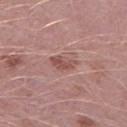{
  "biopsy_status": "not biopsied; imaged during a skin examination",
  "automated_metrics": {
    "cielab_L": 51,
    "cielab_a": 23,
    "cielab_b": 23,
    "vs_skin_darker_L": 9.0,
    "border_irregularity_0_10": 3.5,
    "color_variation_0_10": 3.0,
    "peripheral_color_asymmetry": 1.0,
    "nevus_likeness_0_100": 0,
    "lesion_detection_confidence_0_100": 100
  },
  "lighting": "white-light",
  "site": "left thigh",
  "image": {
    "source": "total-body photography crop",
    "field_of_view_mm": 15
  },
  "patient": {
    "sex": "male",
    "age_approx": 50
  },
  "lesion_size": {
    "long_diameter_mm_approx": 3.5
  }
}